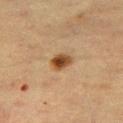Q: Was a biopsy performed?
A: total-body-photography surveillance lesion; no biopsy
Q: Where on the body is the lesion?
A: the leg
Q: Who is the patient?
A: female, in their mid-50s
Q: How was this image acquired?
A: ~15 mm crop, total-body skin-cancer survey
Q: Illumination type?
A: cross-polarized illumination